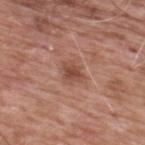Impression:
This lesion was catalogued during total-body skin photography and was not selected for biopsy.
Image and clinical context:
Located on the upper back. A 15 mm close-up extracted from a 3D total-body photography capture. Longest diameter approximately 2.5 mm. An algorithmic analysis of the crop reported a footprint of about 4 mm² and a shape eccentricity near 0.7. The analysis additionally found a mean CIELAB color near L≈47 a*≈24 b*≈28, about 10 CIELAB-L* units darker than the surrounding skin, and a lesion-to-skin contrast of about 7.5 (normalized; higher = more distinct). It also reported a classifier nevus-likeness of about 75/100 and a detector confidence of about 100 out of 100 that the crop contains a lesion. The subject is a male aged 58–62.